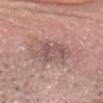Notes:
- workup · no biopsy performed (imaged during a skin exam)
- site · the head or neck
- subject · male, aged approximately 70
- image-analysis metrics · a within-lesion color-variation index near 3.5/10 and radial color variation of about 1; an automated nevus-likeness rating near 0 out of 100 and lesion-presence confidence of about 95/100
- size · ≈5 mm
- acquisition · ~15 mm crop, total-body skin-cancer survey
- lighting · white-light illumination